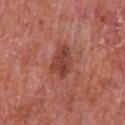Q: Is there a histopathology result?
A: total-body-photography surveillance lesion; no biopsy
Q: What is the lesion's diameter?
A: about 4 mm
Q: What are the patient's age and sex?
A: male, aged 63 to 67
Q: What lighting was used for the tile?
A: white-light illumination
Q: Where on the body is the lesion?
A: the chest
Q: How was this image acquired?
A: ~15 mm crop, total-body skin-cancer survey